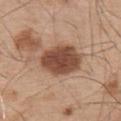{
  "biopsy_status": "not biopsied; imaged during a skin examination",
  "automated_metrics": {
    "border_irregularity_0_10": 1.5,
    "color_variation_0_10": 3.5,
    "peripheral_color_asymmetry": 1.0
  },
  "image": {
    "source": "total-body photography crop",
    "field_of_view_mm": 15
  },
  "patient": {
    "sex": "male",
    "age_approx": 55
  },
  "lesion_size": {
    "long_diameter_mm_approx": 6.0
  },
  "site": "back"
}